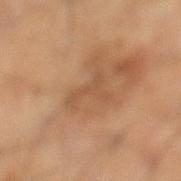Captured during whole-body skin photography for melanoma surveillance; the lesion was not biopsied.
A close-up tile cropped from a whole-body skin photograph, about 15 mm across.
A male subject, aged 58 to 62.
From the right lower leg.